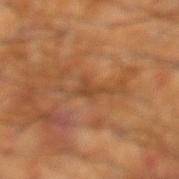This lesion was catalogued during total-body skin photography and was not selected for biopsy. Imaged with cross-polarized lighting. On the left lower leg. A male subject, in their 60s. A close-up tile cropped from a whole-body skin photograph, about 15 mm across. The recorded lesion diameter is about 3 mm.